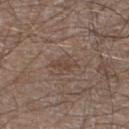Image and clinical context: The lesion is on the left lower leg. A male patient roughly 60 years of age. About 3 mm across. A 15 mm close-up tile from a total-body photography series done for melanoma screening. This is a white-light tile.